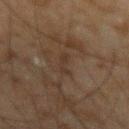biopsy status — total-body-photography surveillance lesion; no biopsy | subject — male, aged around 45 | image source — total-body-photography crop, ~15 mm field of view | body site — the left forearm.